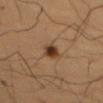Imaged during a routine full-body skin examination; the lesion was not biopsied and no histopathology is available. A male patient aged 48–52. A lesion tile, about 15 mm wide, cut from a 3D total-body photograph. From the left upper arm. Approximately 3.5 mm at its widest. Captured under cross-polarized illumination.